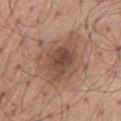<lesion>
<biopsy_status>not biopsied; imaged during a skin examination</biopsy_status>
<lesion_size>
  <long_diameter_mm_approx>6.5</long_diameter_mm_approx>
</lesion_size>
<image>
  <source>total-body photography crop</source>
  <field_of_view_mm>15</field_of_view_mm>
</image>
<patient>
  <sex>male</sex>
  <age_approx>60</age_approx>
</patient>
<site>mid back</site>
<lighting>white-light</lighting>
<automated_metrics>
  <border_irregularity_0_10>3.0</border_irregularity_0_10>
  <nevus_likeness_0_100>60</nevus_likeness_0_100>
  <lesion_detection_confidence_0_100>100</lesion_detection_confidence_0_100>
</automated_metrics>
</lesion>